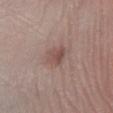<case>
  <biopsy_status>not biopsied; imaged during a skin examination</biopsy_status>
</case>